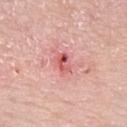The lesion was tiled from a total-body skin photograph and was not biopsied. The lesion's longest dimension is about 2.5 mm. A lesion tile, about 15 mm wide, cut from a 3D total-body photograph. The lesion is on the left upper arm. A female subject aged 58 to 62.